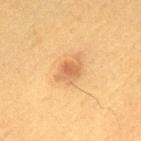Impression: Captured during whole-body skin photography for melanoma surveillance; the lesion was not biopsied. Context: Automated tile analysis of the lesion measured a lesion area of about 7 mm² and an outline eccentricity of about 0.75 (0 = round, 1 = elongated). And it measured a mean CIELAB color near L≈52 a*≈19 b*≈35 and a lesion–skin lightness drop of about 9. And it measured an automated nevus-likeness rating near 80 out of 100. The recorded lesion diameter is about 4 mm. From the upper back. Captured under cross-polarized illumination. The patient is a male aged 48 to 52. A roughly 15 mm field-of-view crop from a total-body skin photograph.